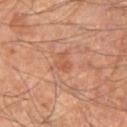Impression: The lesion was photographed on a routine skin check and not biopsied; there is no pathology result. Acquisition and patient details: A close-up tile cropped from a whole-body skin photograph, about 15 mm across. The tile uses cross-polarized illumination. Located on the right thigh. A male patient, in their mid- to late 50s. The lesion-visualizer software estimated a lesion area of about 3.5 mm², an eccentricity of roughly 0.75, and a symmetry-axis asymmetry near 0.45. The software also gave a mean CIELAB color near L≈51 a*≈25 b*≈32, a lesion–skin lightness drop of about 6, and a normalized lesion–skin contrast near 5. The software also gave a border-irregularity index near 4.5/10 and a peripheral color-asymmetry measure near 0.5. And it measured a nevus-likeness score of about 0/100 and lesion-presence confidence of about 100/100.